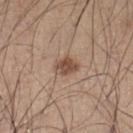Recorded during total-body skin imaging; not selected for excision or biopsy. A male subject approximately 35 years of age. On the leg. The total-body-photography lesion software estimated a footprint of about 5 mm², an outline eccentricity of about 0.65 (0 = round, 1 = elongated), and a shape-asymmetry score of about 0.2 (0 = symmetric). And it measured a mean CIELAB color near L≈49 a*≈19 b*≈28 and about 12 CIELAB-L* units darker than the surrounding skin. It also reported a border-irregularity index near 2/10, a color-variation rating of about 4/10, and a peripheral color-asymmetry measure near 1.5. The software also gave an automated nevus-likeness rating near 85 out of 100 and a detector confidence of about 100 out of 100 that the crop contains a lesion. About 3 mm across. A roughly 15 mm field-of-view crop from a total-body skin photograph. This is a white-light tile.